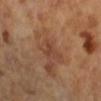A 15 mm close-up extracted from a 3D total-body photography capture.
A female subject, aged 63 to 67.
Located on the left arm.
Approximately 3.5 mm at its widest.
The tile uses cross-polarized illumination.